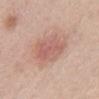Imaged during a routine full-body skin examination; the lesion was not biopsied and no histopathology is available. The total-body-photography lesion software estimated an area of roughly 13 mm², a shape eccentricity near 0.45, and two-axis asymmetry of about 0.2. The analysis additionally found a classifier nevus-likeness of about 25/100 and a lesion-detection confidence of about 100/100. Imaged with white-light lighting. The lesion is located on the mid back. Cropped from a total-body skin-imaging series; the visible field is about 15 mm. About 4.5 mm across. The subject is a male aged 38–42.